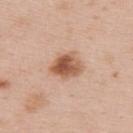Imaged during a routine full-body skin examination; the lesion was not biopsied and no histopathology is available.
A female patient, aged around 35.
The lesion's longest dimension is about 4 mm.
From the upper back.
A region of skin cropped from a whole-body photographic capture, roughly 15 mm wide.
Imaged with white-light lighting.